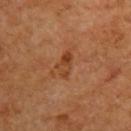| feature | finding |
|---|---|
| notes | no biopsy performed (imaged during a skin exam) |
| image | 15 mm crop, total-body photography |
| anatomic site | the back |
| TBP lesion metrics | an average lesion color of about L≈41 a*≈24 b*≈36 (CIELAB) and a lesion–skin lightness drop of about 8; a border-irregularity index near 3.5/10, a within-lesion color-variation index near 6.5/10, and a peripheral color-asymmetry measure near 2.5 |
| lesion size | ≈3.5 mm |
| subject | male, in their 60s |
| lighting | cross-polarized |